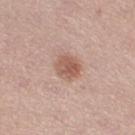This lesion was catalogued during total-body skin photography and was not selected for biopsy.
Longest diameter approximately 3 mm.
A roughly 15 mm field-of-view crop from a total-body skin photograph.
From the leg.
A female subject, approximately 30 years of age.